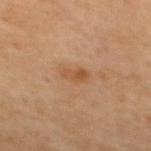Impression: Captured during whole-body skin photography for melanoma surveillance; the lesion was not biopsied. Background: Automated image analysis of the tile measured a footprint of about 3.5 mm². The analysis additionally found an automated nevus-likeness rating near 10 out of 100 and lesion-presence confidence of about 100/100. A female subject approximately 65 years of age. On the upper back. Imaged with cross-polarized lighting. A lesion tile, about 15 mm wide, cut from a 3D total-body photograph. Longest diameter approximately 2.5 mm.